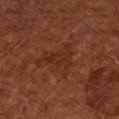Q: Is there a histopathology result?
A: imaged on a skin check; not biopsied
Q: What is the anatomic site?
A: the left forearm
Q: Patient demographics?
A: female, aged around 65
Q: What is the imaging modality?
A: ~15 mm crop, total-body skin-cancer survey